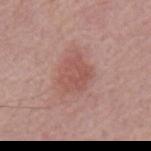Q: Is there a histopathology result?
A: imaged on a skin check; not biopsied
Q: What are the patient's age and sex?
A: male, approximately 65 years of age
Q: What is the imaging modality?
A: ~15 mm crop, total-body skin-cancer survey
Q: Lesion size?
A: ≈4 mm
Q: What lighting was used for the tile?
A: white-light illumination
Q: What is the anatomic site?
A: the mid back